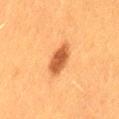Impression: The lesion was tiled from a total-body skin photograph and was not biopsied.